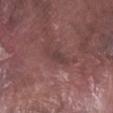{
  "biopsy_status": "not biopsied; imaged during a skin examination",
  "patient": {
    "sex": "male",
    "age_approx": 75
  },
  "site": "right lower leg",
  "lesion_size": {
    "long_diameter_mm_approx": 3.5
  },
  "image": {
    "source": "total-body photography crop",
    "field_of_view_mm": 15
  }
}